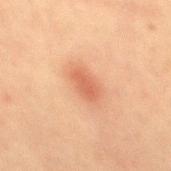Impression:
This lesion was catalogued during total-body skin photography and was not selected for biopsy.
Image and clinical context:
The patient is a male in their 60s. Captured under cross-polarized illumination. Located on the mid back. The total-body-photography lesion software estimated a footprint of about 6 mm² and an outline eccentricity of about 0.85 (0 = round, 1 = elongated). The analysis additionally found a lesion color around L≈52 a*≈24 b*≈31 in CIELAB and roughly 9 lightness units darker than nearby skin. It also reported a border-irregularity index near 1.5/10 and a within-lesion color-variation index near 1.5/10. And it measured lesion-presence confidence of about 100/100. A close-up tile cropped from a whole-body skin photograph, about 15 mm across.